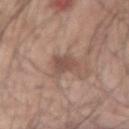No biopsy was performed on this lesion — it was imaged during a full skin examination and was not determined to be concerning. The lesion's longest dimension is about 3 mm. A close-up tile cropped from a whole-body skin photograph, about 15 mm across. The subject is a male roughly 45 years of age. Located on the right forearm.